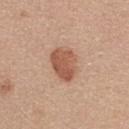Imaged during a routine full-body skin examination; the lesion was not biopsied and no histopathology is available. From the upper back. A region of skin cropped from a whole-body photographic capture, roughly 15 mm wide. Imaged with white-light lighting. Measured at roughly 4 mm in maximum diameter. The total-body-photography lesion software estimated an average lesion color of about L≈55 a*≈23 b*≈31 (CIELAB) and a normalized border contrast of about 8. A female patient, aged 33 to 37.